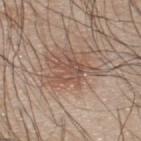workup = catalogued during a skin exam; not biopsied
imaging modality = 15 mm crop, total-body photography
subject = male, in their mid- to late 40s
anatomic site = the upper back